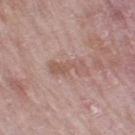Notes:
• image · ~15 mm tile from a whole-body skin photo
• location · the leg
• subject · female, aged approximately 70